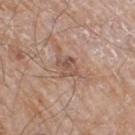Assessment:
Part of a total-body skin-imaging series; this lesion was reviewed on a skin check and was not flagged for biopsy.
Background:
Captured under white-light illumination. Cropped from a total-body skin-imaging series; the visible field is about 15 mm. The subject is a male aged 58 to 62. From the right thigh.